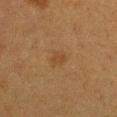Part of a total-body skin-imaging series; this lesion was reviewed on a skin check and was not flagged for biopsy. A 15 mm crop from a total-body photograph taken for skin-cancer surveillance. From the chest. Automated image analysis of the tile measured a lesion color around L≈36 a*≈15 b*≈31 in CIELAB, about 5 CIELAB-L* units darker than the surrounding skin, and a normalized lesion–skin contrast near 5. A female patient, aged 38–42. Measured at roughly 2.5 mm in maximum diameter. Captured under cross-polarized illumination.